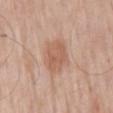Q: Was this lesion biopsied?
A: catalogued during a skin exam; not biopsied
Q: How was this image acquired?
A: ~15 mm crop, total-body skin-cancer survey
Q: How was the tile lit?
A: white-light
Q: How large is the lesion?
A: about 4.5 mm
Q: Where on the body is the lesion?
A: the mid back
Q: What are the patient's age and sex?
A: male, about 60 years old